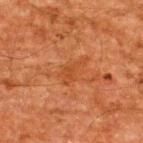Captured during whole-body skin photography for melanoma surveillance; the lesion was not biopsied.
Located on the upper back.
A 15 mm crop from a total-body photograph taken for skin-cancer surveillance.
A male subject aged 58–62.